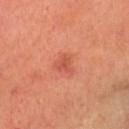Clinical impression:
Part of a total-body skin-imaging series; this lesion was reviewed on a skin check and was not flagged for biopsy.
Image and clinical context:
Cropped from a total-body skin-imaging series; the visible field is about 15 mm. The lesion is located on the head or neck. A female subject in their 40s.